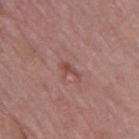Part of a total-body skin-imaging series; this lesion was reviewed on a skin check and was not flagged for biopsy. Captured under white-light illumination. A 15 mm crop from a total-body photograph taken for skin-cancer surveillance. About 2.5 mm across. A male subject aged approximately 55. The lesion is located on the mid back.